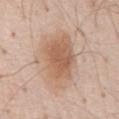Assessment: Recorded during total-body skin imaging; not selected for excision or biopsy. Clinical summary: A male patient aged approximately 70. The lesion's longest dimension is about 7.5 mm. A region of skin cropped from a whole-body photographic capture, roughly 15 mm wide. Automated tile analysis of the lesion measured an area of roughly 24 mm², a shape eccentricity near 0.8, and a symmetry-axis asymmetry near 0.2. And it measured a border-irregularity index near 2.5/10, a color-variation rating of about 4/10, and a peripheral color-asymmetry measure near 1. The lesion is located on the abdomen.